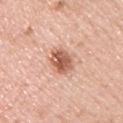Clinical impression:
The lesion was tiled from a total-body skin photograph and was not biopsied.
Acquisition and patient details:
An algorithmic analysis of the crop reported an outline eccentricity of about 0.75 (0 = round, 1 = elongated) and a shape-asymmetry score of about 0.2 (0 = symmetric). It also reported an average lesion color of about L≈60 a*≈24 b*≈31 (CIELAB), about 14 CIELAB-L* units darker than the surrounding skin, and a normalized border contrast of about 9. The software also gave a within-lesion color-variation index near 5/10. This is a white-light tile. Cropped from a total-body skin-imaging series; the visible field is about 15 mm. The recorded lesion diameter is about 4 mm. The lesion is located on the front of the torso. A male subject, aged approximately 25.